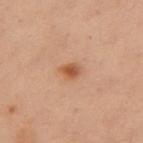Findings:
– notes — imaged on a skin check; not biopsied
– location — the chest
– patient — female, in their mid-50s
– size — ~3 mm (longest diameter)
– acquisition — ~15 mm crop, total-body skin-cancer survey